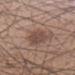Clinical impression: Part of a total-body skin-imaging series; this lesion was reviewed on a skin check and was not flagged for biopsy. Image and clinical context: This is a white-light tile. The total-body-photography lesion software estimated a border-irregularity index near 3/10. The analysis additionally found a classifier nevus-likeness of about 25/100 and a detector confidence of about 100 out of 100 that the crop contains a lesion. A 15 mm close-up tile from a total-body photography series done for melanoma screening. A male patient aged around 55. The lesion's longest dimension is about 5 mm.